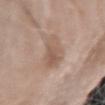Clinical impression:
Recorded during total-body skin imaging; not selected for excision or biopsy.
Background:
A roughly 15 mm field-of-view crop from a total-body skin photograph. The lesion is on the arm. A female patient aged 73 to 77. Longest diameter approximately 5.5 mm.